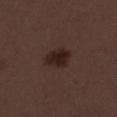  biopsy_status: not biopsied; imaged during a skin examination
  patient:
    sex: female
    age_approx: 50
  site: leg
  image:
    source: total-body photography crop
    field_of_view_mm: 15
  lighting: white-light